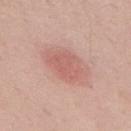Captured during whole-body skin photography for melanoma surveillance; the lesion was not biopsied. Imaged with white-light lighting. Cropped from a whole-body photographic skin survey; the tile spans about 15 mm. The lesion's longest dimension is about 4 mm. Located on the upper back. A male subject aged 68 to 72.